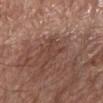Assessment: Part of a total-body skin-imaging series; this lesion was reviewed on a skin check and was not flagged for biopsy. Clinical summary: A 15 mm crop from a total-body photograph taken for skin-cancer surveillance. The lesion's longest dimension is about 4 mm. The tile uses white-light illumination. From the chest. A male patient, aged around 80.